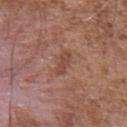Clinical impression: Recorded during total-body skin imaging; not selected for excision or biopsy. Image and clinical context: The lesion is on the upper back. Measured at roughly 3 mm in maximum diameter. Cropped from a total-body skin-imaging series; the visible field is about 15 mm. The tile uses white-light illumination. The subject is a male aged 53 to 57.